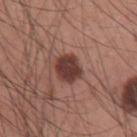workup — no biopsy performed (imaged during a skin exam) | image-analysis metrics — a lesion–skin lightness drop of about 14 and a normalized border contrast of about 11.5; a border-irregularity index near 2/10, a within-lesion color-variation index near 3/10, and a peripheral color-asymmetry measure near 1 | patient — male, aged approximately 35 | image — 15 mm crop, total-body photography | body site — the left upper arm | lighting — white-light illumination | size — ~3 mm (longest diameter).